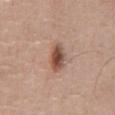Imaged during a routine full-body skin examination; the lesion was not biopsied and no histopathology is available.
A male subject, roughly 75 years of age.
Automated image analysis of the tile measured a footprint of about 6 mm² and two-axis asymmetry of about 0.2. The software also gave a lesion color around L≈49 a*≈21 b*≈28 in CIELAB, about 14 CIELAB-L* units darker than the surrounding skin, and a lesion-to-skin contrast of about 10 (normalized; higher = more distinct). It also reported a border-irregularity rating of about 2/10, internal color variation of about 7 on a 0–10 scale, and radial color variation of about 2.5.
The lesion is on the abdomen.
Measured at roughly 3 mm in maximum diameter.
A region of skin cropped from a whole-body photographic capture, roughly 15 mm wide.
The tile uses white-light illumination.